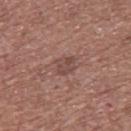The lesion was photographed on a routine skin check and not biopsied; there is no pathology result.
This is a white-light tile.
About 2.5 mm across.
A male subject aged approximately 60.
Automated image analysis of the tile measured a lesion area of about 4.5 mm² and a shape eccentricity near 0.6.
A 15 mm close-up extracted from a 3D total-body photography capture.
Located on the upper back.